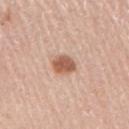The lesion was tiled from a total-body skin photograph and was not biopsied. A male patient, aged 58–62. A roughly 15 mm field-of-view crop from a total-body skin photograph. On the right upper arm. The total-body-photography lesion software estimated a lesion color around L≈58 a*≈22 b*≈31 in CIELAB and a lesion-to-skin contrast of about 9 (normalized; higher = more distinct). And it measured an automated nevus-likeness rating near 100 out of 100 and lesion-presence confidence of about 100/100.